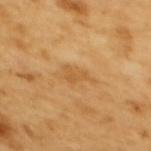– diameter — about 3.5 mm
– body site — the back
– image — total-body-photography crop, ~15 mm field of view
– patient — female, about 55 years old
– image-analysis metrics — a lesion-detection confidence of about 100/100
– illumination — cross-polarized illumination